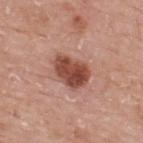| feature | finding |
|---|---|
| biopsy status | total-body-photography surveillance lesion; no biopsy |
| diameter | ~4.5 mm (longest diameter) |
| location | the back |
| lighting | white-light illumination |
| image source | ~15 mm crop, total-body skin-cancer survey |
| patient | male, in their mid-70s |